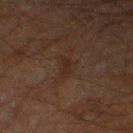Q: Was this lesion biopsied?
A: no biopsy performed (imaged during a skin exam)
Q: Who is the patient?
A: male, aged 58 to 62
Q: How was the tile lit?
A: cross-polarized
Q: What is the imaging modality?
A: ~15 mm crop, total-body skin-cancer survey
Q: Where on the body is the lesion?
A: the left thigh